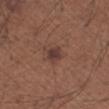Q: Lesion size?
A: ~2.5 mm (longest diameter)
Q: Where on the body is the lesion?
A: the left forearm
Q: What is the imaging modality?
A: ~15 mm crop, total-body skin-cancer survey
Q: How was the tile lit?
A: white-light
Q: Patient demographics?
A: male, aged around 65